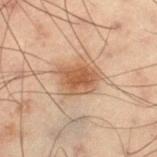| key | value |
|---|---|
| follow-up | total-body-photography surveillance lesion; no biopsy |
| body site | the left thigh |
| size | ~4.5 mm (longest diameter) |
| automated metrics | a nevus-likeness score of about 90/100 |
| lighting | cross-polarized illumination |
| subject | male, about 55 years old |
| image | 15 mm crop, total-body photography |